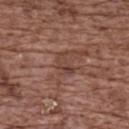Notes:
– follow-up — no biopsy performed (imaged during a skin exam)
– subject — female, aged around 75
– TBP lesion metrics — an area of roughly 4 mm², a shape eccentricity near 0.85, and two-axis asymmetry of about 0.4; a border-irregularity index near 4/10 and a color-variation rating of about 5/10; a classifier nevus-likeness of about 0/100 and a lesion-detection confidence of about 55/100
– image — ~15 mm tile from a whole-body skin photo
– lesion diameter — ~3 mm (longest diameter)
– location — the upper back